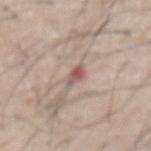Q: Was a biopsy performed?
A: imaged on a skin check; not biopsied